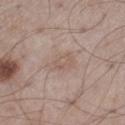The lesion was photographed on a routine skin check and not biopsied; there is no pathology result. A close-up tile cropped from a whole-body skin photograph, about 15 mm across. This is a white-light tile. A male subject in their mid-50s. The recorded lesion diameter is about 3.5 mm. On the left thigh. An algorithmic analysis of the crop reported a footprint of about 6.5 mm², a shape eccentricity near 0.75, and a shape-asymmetry score of about 0.35 (0 = symmetric). The analysis additionally found a lesion color around L≈57 a*≈15 b*≈24 in CIELAB, roughly 6 lightness units darker than nearby skin, and a normalized border contrast of about 4.5.